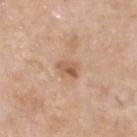Q: Was this lesion biopsied?
A: no biopsy performed (imaged during a skin exam)